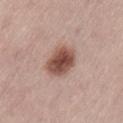{"biopsy_status": "not biopsied; imaged during a skin examination", "lesion_size": {"long_diameter_mm_approx": 4.0}, "site": "back", "image": {"source": "total-body photography crop", "field_of_view_mm": 15}, "lighting": "white-light", "patient": {"sex": "male", "age_approx": 65}}